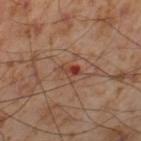{
  "biopsy_status": "not biopsied; imaged during a skin examination",
  "patient": {
    "sex": "male",
    "age_approx": 55
  },
  "site": "left thigh",
  "image": {
    "source": "total-body photography crop",
    "field_of_view_mm": 15
  }
}